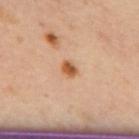workup — total-body-photography surveillance lesion; no biopsy
tile lighting — cross-polarized illumination
lesion diameter — about 2 mm
acquisition — total-body-photography crop, ~15 mm field of view
body site — the upper back
subject — female, approximately 40 years of age